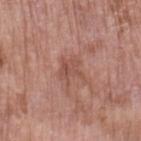Imaged during a routine full-body skin examination; the lesion was not biopsied and no histopathology is available.
Automated image analysis of the tile measured an eccentricity of roughly 0.5 and a shape-asymmetry score of about 0.45 (0 = symmetric). And it measured a lesion color around L≈51 a*≈23 b*≈27 in CIELAB, a lesion–skin lightness drop of about 7, and a normalized border contrast of about 5.5. The software also gave an automated nevus-likeness rating near 0 out of 100 and a lesion-detection confidence of about 100/100.
A female subject, about 70 years old.
Approximately 3.5 mm at its widest.
Captured under white-light illumination.
A region of skin cropped from a whole-body photographic capture, roughly 15 mm wide.
Located on the left upper arm.